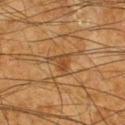Impression:
The lesion was photographed on a routine skin check and not biopsied; there is no pathology result.
Image and clinical context:
A male patient, in their mid- to late 60s. A 15 mm close-up tile from a total-body photography series done for melanoma screening. The lesion's longest dimension is about 3 mm. Imaged with cross-polarized lighting. From the right lower leg.